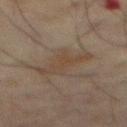This lesion was catalogued during total-body skin photography and was not selected for biopsy. On the abdomen. A male patient about 70 years old. A roughly 15 mm field-of-view crop from a total-body skin photograph.